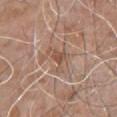<record>
<lesion_size>
  <long_diameter_mm_approx>3.5</long_diameter_mm_approx>
</lesion_size>
<site>chest</site>
<image>
  <source>total-body photography crop</source>
  <field_of_view_mm>15</field_of_view_mm>
</image>
<automated_metrics>
  <eccentricity>0.7</eccentricity>
  <shape_asymmetry>0.7</shape_asymmetry>
  <border_irregularity_0_10>7.5</border_irregularity_0_10>
  <nevus_likeness_0_100>0</nevus_likeness_0_100>
</automated_metrics>
<patient>
  <sex>male</sex>
  <age_approx>80</age_approx>
</patient>
<lighting>white-light</lighting>
</record>